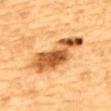workup = catalogued during a skin exam; not biopsied
patient = male, aged around 85 or older
anatomic site = the back
lesion size = ~8.5 mm (longest diameter)
imaging modality = ~15 mm crop, total-body skin-cancer survey
illumination = cross-polarized illumination